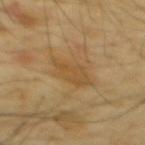Case summary:
* workup — catalogued during a skin exam; not biopsied
* patient — male, about 65 years old
* acquisition — total-body-photography crop, ~15 mm field of view
* site — the mid back